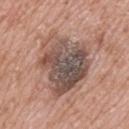Acquisition and patient details: Captured under white-light illumination. Located on the upper back. An algorithmic analysis of the crop reported an outline eccentricity of about 0.65 (0 = round, 1 = elongated) and a symmetry-axis asymmetry near 0.3. And it measured a border-irregularity rating of about 4/10, a within-lesion color-variation index near 9/10, and a peripheral color-asymmetry measure near 3. The analysis additionally found a nevus-likeness score of about 5/100. A male patient in their mid- to late 70s. The lesion's longest dimension is about 7.5 mm. A 15 mm close-up extracted from a 3D total-body photography capture.